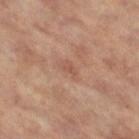The lesion was tiled from a total-body skin photograph and was not biopsied.
Captured under cross-polarized illumination.
The patient is a female aged approximately 65.
A lesion tile, about 15 mm wide, cut from a 3D total-body photograph.
The lesion is on the left leg.
The lesion-visualizer software estimated an area of roughly 3.5 mm² and two-axis asymmetry of about 0.4. The analysis additionally found a nevus-likeness score of about 0/100 and lesion-presence confidence of about 100/100.
The lesion's longest dimension is about 3 mm.